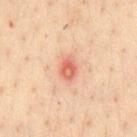* workup: imaged on a skin check; not biopsied
* lesion diameter: ≈3 mm
* tile lighting: cross-polarized
* patient: male, aged 33 to 37
* image: ~15 mm tile from a whole-body skin photo
* site: the chest